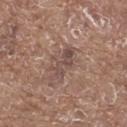Recorded during total-body skin imaging; not selected for excision or biopsy.
Approximately 4 mm at its widest.
Cropped from a whole-body photographic skin survey; the tile spans about 15 mm.
A male subject, roughly 80 years of age.
From the upper back.